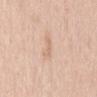Imaged during a routine full-body skin examination; the lesion was not biopsied and no histopathology is available. Captured under white-light illumination. Cropped from a whole-body photographic skin survey; the tile spans about 15 mm. The lesion is located on the mid back. An algorithmic analysis of the crop reported a lesion area of about 2.5 mm², a shape eccentricity near 0.9, and a symmetry-axis asymmetry near 0.4. The analysis additionally found a lesion–skin lightness drop of about 7 and a normalized border contrast of about 4.5. And it measured peripheral color asymmetry of about 0. It also reported a classifier nevus-likeness of about 0/100 and a detector confidence of about 100 out of 100 that the crop contains a lesion. The subject is a female approximately 70 years of age.